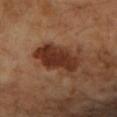workup: imaged on a skin check; not biopsied | image: ~15 mm tile from a whole-body skin photo | diameter: ~6 mm (longest diameter) | TBP lesion metrics: an area of roughly 17 mm² and a symmetry-axis asymmetry near 0.2; a mean CIELAB color near L≈34 a*≈23 b*≈30, a lesion–skin lightness drop of about 13, and a lesion-to-skin contrast of about 11.5 (normalized; higher = more distinct); border irregularity of about 3 on a 0–10 scale, a color-variation rating of about 4/10, and a peripheral color-asymmetry measure near 1 | patient: female, aged approximately 70 | illumination: cross-polarized | anatomic site: the left forearm.